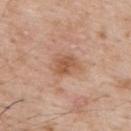This lesion was catalogued during total-body skin photography and was not selected for biopsy. The lesion's longest dimension is about 3.5 mm. Imaged with white-light lighting. A roughly 15 mm field-of-view crop from a total-body skin photograph. Located on the upper back. A male subject, aged 58–62.